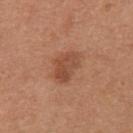follow-up: catalogued during a skin exam; not biopsied
location: the right upper arm
automated metrics: an area of roughly 8 mm², an outline eccentricity of about 0.75 (0 = round, 1 = elongated), and a symmetry-axis asymmetry near 0.25; a normalized border contrast of about 6.5; a border-irregularity index near 3/10, a color-variation rating of about 3.5/10, and a peripheral color-asymmetry measure near 1
subject: female, aged 23 to 27
acquisition: ~15 mm tile from a whole-body skin photo
lesion size: ~4 mm (longest diameter)